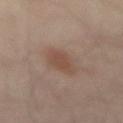No biopsy was performed on this lesion — it was imaged during a full skin examination and was not determined to be concerning. The subject is roughly 55 years of age. The tile uses cross-polarized illumination. The lesion-visualizer software estimated an area of roughly 7.5 mm² and two-axis asymmetry of about 0.2. And it measured a border-irregularity index near 2.5/10, a within-lesion color-variation index near 2/10, and peripheral color asymmetry of about 1. A close-up tile cropped from a whole-body skin photograph, about 15 mm across. Located on the mid back. The lesion's longest dimension is about 4 mm.